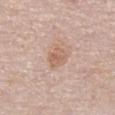Q: Was this lesion biopsied?
A: total-body-photography surveillance lesion; no biopsy